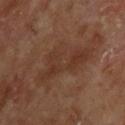The lesion was tiled from a total-body skin photograph and was not biopsied. The tile uses cross-polarized illumination. A 15 mm crop from a total-body photograph taken for skin-cancer surveillance. From the upper back. A male patient roughly 70 years of age.